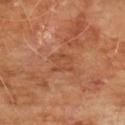Case summary:
– notes · catalogued during a skin exam; not biopsied
– lesion diameter · ≈3 mm
– illumination · cross-polarized illumination
– subject · male, aged 58 to 62
– image source · ~15 mm tile from a whole-body skin photo
– body site · the chest